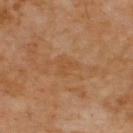Clinical impression:
No biopsy was performed on this lesion — it was imaged during a full skin examination and was not determined to be concerning.
Clinical summary:
This is a cross-polarized tile. The lesion's longest dimension is about 2.5 mm. Cropped from a whole-body photographic skin survey; the tile spans about 15 mm. Located on the upper back. A male patient, aged around 70.